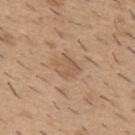{
  "biopsy_status": "not biopsied; imaged during a skin examination",
  "automated_metrics": {
    "cielab_L": 56,
    "cielab_a": 17,
    "cielab_b": 32,
    "vs_skin_darker_L": 7.0,
    "vs_skin_contrast_norm": 5.0
  },
  "image": {
    "source": "total-body photography crop",
    "field_of_view_mm": 15
  },
  "lesion_size": {
    "long_diameter_mm_approx": 2.5
  },
  "patient": {
    "sex": "male",
    "age_approx": 40
  },
  "site": "upper back"
}